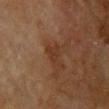Captured during whole-body skin photography for melanoma surveillance; the lesion was not biopsied.
The lesion is on the chest.
The lesion's longest dimension is about 3.5 mm.
A female subject, in their 80s.
The total-body-photography lesion software estimated a peripheral color-asymmetry measure near 0.5. The software also gave a lesion-detection confidence of about 100/100.
A lesion tile, about 15 mm wide, cut from a 3D total-body photograph.